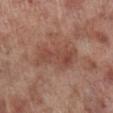| key | value |
|---|---|
| biopsy status | no biopsy performed (imaged during a skin exam) |
| location | the right lower leg |
| lighting | white-light |
| image source | 15 mm crop, total-body photography |
| patient | male, roughly 70 years of age |